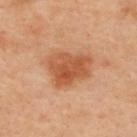Clinical impression: No biopsy was performed on this lesion — it was imaged during a full skin examination and was not determined to be concerning. Context: The total-body-photography lesion software estimated an average lesion color of about L≈56 a*≈27 b*≈38 (CIELAB), a lesion–skin lightness drop of about 12, and a normalized lesion–skin contrast near 8. A 15 mm close-up extracted from a 3D total-body photography capture. This is a cross-polarized tile. On the upper back. A female subject, aged 38 to 42. The recorded lesion diameter is about 5.5 mm.